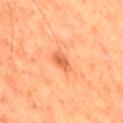biopsy status = no biopsy performed (imaged during a skin exam) | location = the right thigh | automated lesion analysis = a footprint of about 3.5 mm² and a shape eccentricity near 0.75; a classifier nevus-likeness of about 45/100 and lesion-presence confidence of about 100/100 | diameter = about 2.5 mm | patient = male, approximately 65 years of age | acquisition = ~15 mm crop, total-body skin-cancer survey | lighting = cross-polarized.